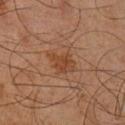• follow-up: catalogued during a skin exam; not biopsied
• illumination: cross-polarized illumination
• lesion diameter: ≈3.5 mm
• image-analysis metrics: a lesion area of about 6 mm² and a shape eccentricity near 0.75; roughly 6 lightness units darker than nearby skin and a normalized lesion–skin contrast near 6.5; border irregularity of about 3 on a 0–10 scale, a within-lesion color-variation index near 2/10, and peripheral color asymmetry of about 0.5; an automated nevus-likeness rating near 10 out of 100 and a lesion-detection confidence of about 100/100
• patient: male, in their mid-60s
• image: ~15 mm crop, total-body skin-cancer survey
• anatomic site: the right lower leg